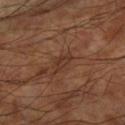notes — no biopsy performed (imaged during a skin exam)
image source — total-body-photography crop, ~15 mm field of view
subject — male, in their 60s
lesion size — ~3 mm (longest diameter)
tile lighting — cross-polarized
body site — the arm